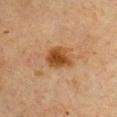workup = total-body-photography surveillance lesion; no biopsy
illumination = cross-polarized illumination
diameter = ≈4 mm
acquisition = total-body-photography crop, ~15 mm field of view
anatomic site = the chest
subject = female, in their mid- to late 50s